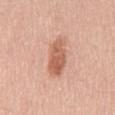Part of a total-body skin-imaging series; this lesion was reviewed on a skin check and was not flagged for biopsy. This is a white-light tile. A lesion tile, about 15 mm wide, cut from a 3D total-body photograph. On the mid back. The subject is a male aged 58–62.